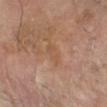patient — male, in their mid-60s | lesion diameter — about 3 mm | illumination — cross-polarized illumination | imaging modality — total-body-photography crop, ~15 mm field of view | automated lesion analysis — a shape eccentricity near 0.85 and a shape-asymmetry score of about 0.3 (0 = symmetric); an average lesion color of about L≈50 a*≈19 b*≈30 (CIELAB), a lesion–skin lightness drop of about 5, and a normalized border contrast of about 4.5; an automated nevus-likeness rating near 0 out of 100 and lesion-presence confidence of about 100/100 | location — the right forearm.